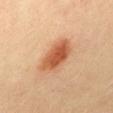notes — catalogued during a skin exam; not biopsied | imaging modality — total-body-photography crop, ~15 mm field of view | tile lighting — cross-polarized illumination | TBP lesion metrics — a footprint of about 13 mm², a shape eccentricity near 0.8, and two-axis asymmetry of about 0.2; about 13 CIELAB-L* units darker than the surrounding skin and a lesion-to-skin contrast of about 9 (normalized; higher = more distinct) | location — the back | lesion size — about 5 mm | patient — female, aged 33–37.